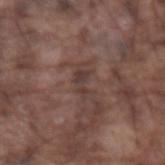{
  "biopsy_status": "not biopsied; imaged during a skin examination",
  "patient": {
    "sex": "male",
    "age_approx": 75
  },
  "lesion_size": {
    "long_diameter_mm_approx": 2.5
  },
  "image": {
    "source": "total-body photography crop",
    "field_of_view_mm": 15
  },
  "lighting": "white-light",
  "site": "arm",
  "automated_metrics": {
    "area_mm2_approx": 3.5,
    "eccentricity": 0.85,
    "shape_asymmetry": 0.5,
    "cielab_L": 37,
    "cielab_a": 16,
    "cielab_b": 19,
    "vs_skin_darker_L": 7.0,
    "vs_skin_contrast_norm": 6.5,
    "nevus_likeness_0_100": 0,
    "lesion_detection_confidence_0_100": 75
  }
}